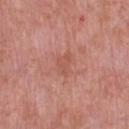Recorded during total-body skin imaging; not selected for excision or biopsy.
A male subject, in their 50s.
From the front of the torso.
Imaged with white-light lighting.
A 15 mm close-up tile from a total-body photography series done for melanoma screening.
The recorded lesion diameter is about 3 mm.